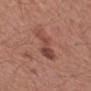  image:
    source: total-body photography crop
    field_of_view_mm: 15
  site: left forearm
  patient:
    sex: female
    age_approx: 50